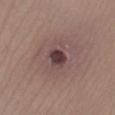Q: Is there a histopathology result?
A: total-body-photography surveillance lesion; no biopsy
Q: Illumination type?
A: white-light
Q: What are the patient's age and sex?
A: female, approximately 35 years of age
Q: What is the lesion's diameter?
A: ≈2.5 mm
Q: Where on the body is the lesion?
A: the right lower leg
Q: How was this image acquired?
A: 15 mm crop, total-body photography
Q: What did automated image analysis measure?
A: an area of roughly 4.5 mm², an eccentricity of roughly 0.6, and two-axis asymmetry of about 0.2